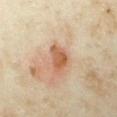Background: Cropped from a whole-body photographic skin survey; the tile spans about 15 mm. On the right forearm. A female patient aged 33 to 37.